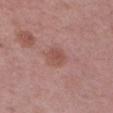No biopsy was performed on this lesion — it was imaged during a full skin examination and was not determined to be concerning. Approximately 2.5 mm at its widest. Cropped from a total-body skin-imaging series; the visible field is about 15 mm. Automated tile analysis of the lesion measured an automated nevus-likeness rating near 30 out of 100 and a detector confidence of about 100 out of 100 that the crop contains a lesion. The lesion is located on the left upper arm. Imaged with white-light lighting. The patient is a male about 50 years old.